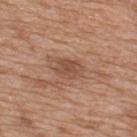The lesion was photographed on a routine skin check and not biopsied; there is no pathology result.
About 3.5 mm across.
The subject is a male aged approximately 50.
Imaged with white-light lighting.
Automated tile analysis of the lesion measured an average lesion color of about L≈50 a*≈21 b*≈29 (CIELAB) and a normalized lesion–skin contrast near 6.5. The analysis additionally found border irregularity of about 2 on a 0–10 scale, a color-variation rating of about 2.5/10, and a peripheral color-asymmetry measure near 0.5. It also reported a detector confidence of about 100 out of 100 that the crop contains a lesion.
A region of skin cropped from a whole-body photographic capture, roughly 15 mm wide.
From the upper back.